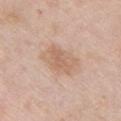Clinical impression: Captured during whole-body skin photography for melanoma surveillance; the lesion was not biopsied. Clinical summary: A 15 mm crop from a total-body photograph taken for skin-cancer surveillance. The subject is a female in their mid- to late 60s. Imaged with white-light lighting. The lesion-visualizer software estimated a mean CIELAB color near L≈63 a*≈18 b*≈30, about 8 CIELAB-L* units darker than the surrounding skin, and a lesion-to-skin contrast of about 6 (normalized; higher = more distinct). And it measured a border-irregularity rating of about 2/10, a within-lesion color-variation index near 3/10, and radial color variation of about 1. The lesion is on the chest. Measured at roughly 4.5 mm in maximum diameter.